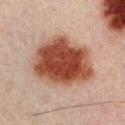{"biopsy_status": "not biopsied; imaged during a skin examination", "lesion_size": {"long_diameter_mm_approx": 8.0}, "patient": {"sex": "male", "age_approx": 30}, "image": {"source": "total-body photography crop", "field_of_view_mm": 15}, "site": "chest", "lighting": "cross-polarized"}